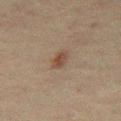Clinical impression:
Recorded during total-body skin imaging; not selected for excision or biopsy.
Image and clinical context:
Cropped from a whole-body photographic skin survey; the tile spans about 15 mm. A male patient roughly 50 years of age. Approximately 2.5 mm at its widest. From the abdomen.